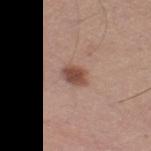The lesion was photographed on a routine skin check and not biopsied; there is no pathology result. Automated tile analysis of the lesion measured a footprint of about 5 mm², an eccentricity of roughly 0.7, and two-axis asymmetry of about 0.15. It also reported an average lesion color of about L≈49 a*≈21 b*≈26 (CIELAB), about 13 CIELAB-L* units darker than the surrounding skin, and a lesion-to-skin contrast of about 9 (normalized; higher = more distinct). The software also gave a nevus-likeness score of about 95/100 and a detector confidence of about 100 out of 100 that the crop contains a lesion. The subject is a male about 50 years old. The recorded lesion diameter is about 3 mm. Located on the right thigh. Cropped from a whole-body photographic skin survey; the tile spans about 15 mm.